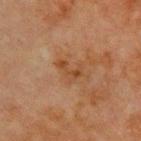automated lesion analysis: a lesion area of about 3.5 mm², a shape eccentricity near 0.85, and a shape-asymmetry score of about 0.6 (0 = symmetric); a classifier nevus-likeness of about 0/100 and a lesion-detection confidence of about 100/100 | acquisition: 15 mm crop, total-body photography | patient: male, aged around 70 | lighting: cross-polarized | lesion diameter: ~3 mm (longest diameter) | body site: the chest.